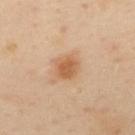Impression:
This lesion was catalogued during total-body skin photography and was not selected for biopsy.
Background:
A 15 mm close-up tile from a total-body photography series done for melanoma screening. A female patient, about 40 years old. On the upper back. The recorded lesion diameter is about 3 mm.